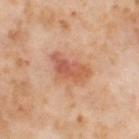Part of a total-body skin-imaging series; this lesion was reviewed on a skin check and was not flagged for biopsy. A female subject, in their mid- to late 50s. Cropped from a whole-body photographic skin survey; the tile spans about 15 mm. The lesion's longest dimension is about 4.5 mm. From the left thigh. Automated image analysis of the tile measured an outline eccentricity of about 0.8 (0 = round, 1 = elongated) and two-axis asymmetry of about 0.35. The software also gave a border-irregularity index near 4/10, a within-lesion color-variation index near 5.5/10, and radial color variation of about 2.